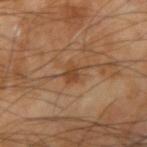Impression: No biopsy was performed on this lesion — it was imaged during a full skin examination and was not determined to be concerning. Acquisition and patient details: This image is a 15 mm lesion crop taken from a total-body photograph. Captured under cross-polarized illumination. On the right upper arm. About 3.5 mm across. A male patient, about 65 years old.